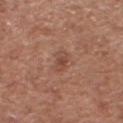The lesion was tiled from a total-body skin photograph and was not biopsied. A male subject about 75 years old. From the chest. The total-body-photography lesion software estimated a footprint of about 4 mm², an eccentricity of roughly 0.8, and a shape-asymmetry score of about 0.2 (0 = symmetric). And it measured about 7 CIELAB-L* units darker than the surrounding skin and a normalized border contrast of about 5.5. It also reported border irregularity of about 2 on a 0–10 scale, a color-variation rating of about 3/10, and radial color variation of about 1. The software also gave a classifier nevus-likeness of about 0/100 and a lesion-detection confidence of about 100/100. A 15 mm crop from a total-body photograph taken for skin-cancer surveillance. Imaged with white-light lighting.